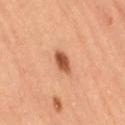workup=catalogued during a skin exam; not biopsied | size=about 3 mm | image source=~15 mm crop, total-body skin-cancer survey | body site=the right thigh | tile lighting=cross-polarized illumination | automated lesion analysis=a footprint of about 4.5 mm², a shape eccentricity near 0.75, and two-axis asymmetry of about 0.15 | patient=female, aged 58 to 62.